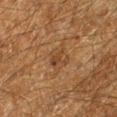Findings:
• notes: total-body-photography surveillance lesion; no biopsy
• location: the left lower leg
• diameter: ≈2.5 mm
• subject: male, aged 58 to 62
• lighting: cross-polarized illumination
• image source: 15 mm crop, total-body photography